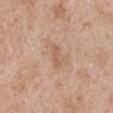Case summary:
- workup — imaged on a skin check; not biopsied